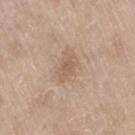Impression: Captured during whole-body skin photography for melanoma surveillance; the lesion was not biopsied. Background: Imaged with white-light lighting. A lesion tile, about 15 mm wide, cut from a 3D total-body photograph. Longest diameter approximately 3 mm. Located on the right thigh. A female patient, in their mid-70s. The total-body-photography lesion software estimated an average lesion color of about L≈58 a*≈17 b*≈28 (CIELAB), roughly 7 lightness units darker than nearby skin, and a normalized border contrast of about 5.